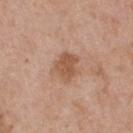biopsy status: no biopsy performed (imaged during a skin exam)
patient: male, aged approximately 55
tile lighting: white-light illumination
site: the upper back
imaging modality: ~15 mm tile from a whole-body skin photo